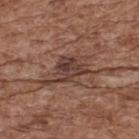biopsy status = catalogued during a skin exam; not biopsied | site = the back | patient = male, aged approximately 75 | imaging modality = total-body-photography crop, ~15 mm field of view.